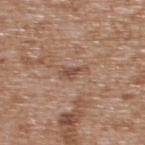{
  "biopsy_status": "not biopsied; imaged during a skin examination",
  "automated_metrics": {
    "area_mm2_approx": 3.0,
    "eccentricity": 0.9,
    "shape_asymmetry": 0.45,
    "cielab_L": 49,
    "cielab_a": 19,
    "cielab_b": 28,
    "vs_skin_darker_L": 9.0,
    "vs_skin_contrast_norm": 6.5,
    "nevus_likeness_0_100": 0
  },
  "lighting": "white-light",
  "site": "upper back",
  "lesion_size": {
    "long_diameter_mm_approx": 3.0
  },
  "patient": {
    "sex": "male",
    "age_approx": 65
  },
  "image": {
    "source": "total-body photography crop",
    "field_of_view_mm": 15
  }
}